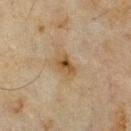Impression:
The lesion was tiled from a total-body skin photograph and was not biopsied.
Clinical summary:
A male patient, in their 70s. A close-up tile cropped from a whole-body skin photograph, about 15 mm across. The total-body-photography lesion software estimated a classifier nevus-likeness of about 15/100 and lesion-presence confidence of about 100/100. The lesion's longest dimension is about 3 mm. The lesion is located on the chest. This is a cross-polarized tile.